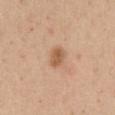notes — total-body-photography surveillance lesion; no biopsy | patient — female, aged approximately 30 | anatomic site — the abdomen | image — ~15 mm tile from a whole-body skin photo | automated metrics — an eccentricity of roughly 0.75 and two-axis asymmetry of about 0.2; an average lesion color of about L≈58 a*≈21 b*≈35 (CIELAB) and a lesion–skin lightness drop of about 11; internal color variation of about 2 on a 0–10 scale; a classifier nevus-likeness of about 55/100 and lesion-presence confidence of about 100/100 | lighting — white-light | lesion size — about 2.5 mm.